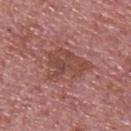Q: Is there a histopathology result?
A: imaged on a skin check; not biopsied
Q: How large is the lesion?
A: ~5.5 mm (longest diameter)
Q: What lighting was used for the tile?
A: white-light illumination
Q: What are the patient's age and sex?
A: male, aged around 75
Q: How was this image acquired?
A: ~15 mm tile from a whole-body skin photo
Q: Automated lesion metrics?
A: an area of roughly 16 mm² and two-axis asymmetry of about 0.35; an average lesion color of about L≈47 a*≈24 b*≈25 (CIELAB), about 8 CIELAB-L* units darker than the surrounding skin, and a lesion-to-skin contrast of about 6.5 (normalized; higher = more distinct)
Q: Lesion location?
A: the upper back